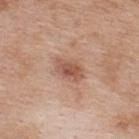Impression: This lesion was catalogued during total-body skin photography and was not selected for biopsy. Context: Cropped from a whole-body photographic skin survey; the tile spans about 15 mm. Approximately 3.5 mm at its widest. Captured under white-light illumination. The subject is a female about 40 years old. The lesion is on the upper back.